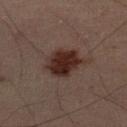A male patient about 50 years old. The lesion is located on the right lower leg. A lesion tile, about 15 mm wide, cut from a 3D total-body photograph. Captured under cross-polarized illumination. Approximately 4 mm at its widest.